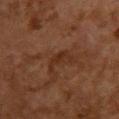notes = imaged on a skin check; not biopsied | anatomic site = the upper back | subject = female, aged 58 to 62 | image source = ~15 mm tile from a whole-body skin photo | tile lighting = cross-polarized | diameter = ~3.5 mm (longest diameter).